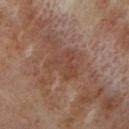{
  "biopsy_status": "not biopsied; imaged during a skin examination",
  "site": "left lower leg",
  "image": {
    "source": "total-body photography crop",
    "field_of_view_mm": 15
  },
  "patient": {
    "sex": "female",
    "age_approx": 50
  }
}